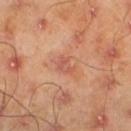On the left lower leg.
About 2.5 mm across.
The patient is a male aged 43 to 47.
A 15 mm crop from a total-body photograph taken for skin-cancer surveillance.